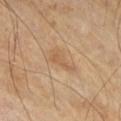{"biopsy_status": "not biopsied; imaged during a skin examination", "image": {"source": "total-body photography crop", "field_of_view_mm": 15}, "lighting": "cross-polarized", "site": "right thigh", "lesion_size": {"long_diameter_mm_approx": 3.5}, "patient": {"sex": "male", "age_approx": 70}}